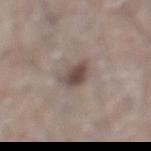workup = imaged on a skin check; not biopsied
anatomic site = the abdomen
lesion diameter = ~3 mm (longest diameter)
subject = male, in their mid-70s
image-analysis metrics = a shape eccentricity near 0.65 and two-axis asymmetry of about 0.25; an average lesion color of about L≈47 a*≈13 b*≈19 (CIELAB), a lesion–skin lightness drop of about 12, and a normalized lesion–skin contrast near 9; a border-irregularity rating of about 2.5/10, a color-variation rating of about 3/10, and a peripheral color-asymmetry measure near 1
lighting = white-light
imaging modality = 15 mm crop, total-body photography